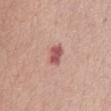No biopsy was performed on this lesion — it was imaged during a full skin examination and was not determined to be concerning.
Captured under white-light illumination.
A 15 mm close-up extracted from a 3D total-body photography capture.
On the chest.
A female subject, aged 63 to 67.
The lesion's longest dimension is about 3 mm.